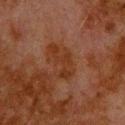Part of a total-body skin-imaging series; this lesion was reviewed on a skin check and was not flagged for biopsy.
The lesion is on the upper back.
Imaged with cross-polarized lighting.
A male patient approximately 80 years of age.
A close-up tile cropped from a whole-body skin photograph, about 15 mm across.
The lesion's longest dimension is about 5 mm.